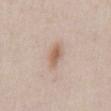This lesion was catalogued during total-body skin photography and was not selected for biopsy.
Measured at roughly 3 mm in maximum diameter.
Imaged with white-light lighting.
The lesion-visualizer software estimated an area of roughly 4.5 mm², an eccentricity of roughly 0.85, and a symmetry-axis asymmetry near 0.25. The software also gave an average lesion color of about L≈61 a*≈17 b*≈28 (CIELAB), a lesion–skin lightness drop of about 10, and a normalized lesion–skin contrast near 7.5. The software also gave a border-irregularity index near 2.5/10 and a color-variation rating of about 2.5/10.
Located on the chest.
A roughly 15 mm field-of-view crop from a total-body skin photograph.
A male patient, aged around 40.